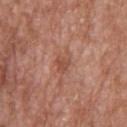notes: catalogued during a skin exam; not biopsied
location: the upper back
acquisition: ~15 mm crop, total-body skin-cancer survey
lesion diameter: ≈3 mm
subject: female, aged around 75
illumination: white-light
automated lesion analysis: a footprint of about 4 mm², an outline eccentricity of about 0.7 (0 = round, 1 = elongated), and a shape-asymmetry score of about 0.4 (0 = symmetric); an average lesion color of about L≈50 a*≈24 b*≈30 (CIELAB) and roughly 8 lightness units darker than nearby skin